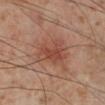<lesion>
  <biopsy_status>not biopsied; imaged during a skin examination</biopsy_status>
</lesion>